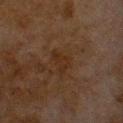biopsy_status: not biopsied; imaged during a skin examination
site: chest
lighting: cross-polarized
lesion_size:
  long_diameter_mm_approx: 3.0
patient:
  sex: male
  age_approx: 80
image:
  source: total-body photography crop
  field_of_view_mm: 15